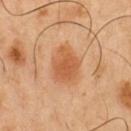{"biopsy_status": "not biopsied; imaged during a skin examination", "image": {"source": "total-body photography crop", "field_of_view_mm": 15}, "automated_metrics": {"color_variation_0_10": 3.0, "peripheral_color_asymmetry": 1.0, "lesion_detection_confidence_0_100": 100}, "patient": {"sex": "male", "age_approx": 50}, "lesion_size": {"long_diameter_mm_approx": 4.5}, "site": "chest", "lighting": "cross-polarized"}